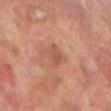Assessment: The lesion was tiled from a total-body skin photograph and was not biopsied.